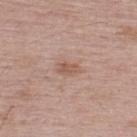Clinical impression:
No biopsy was performed on this lesion — it was imaged during a full skin examination and was not determined to be concerning.
Acquisition and patient details:
Longest diameter approximately 2.5 mm. A roughly 15 mm field-of-view crop from a total-body skin photograph. The total-body-photography lesion software estimated a footprint of about 3.5 mm², an outline eccentricity of about 0.8 (0 = round, 1 = elongated), and a shape-asymmetry score of about 0.25 (0 = symmetric). It also reported a classifier nevus-likeness of about 0/100 and lesion-presence confidence of about 100/100. The subject is a male in their mid- to late 60s. From the back.